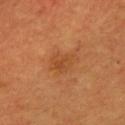Part of a total-body skin-imaging series; this lesion was reviewed on a skin check and was not flagged for biopsy. Automated tile analysis of the lesion measured an average lesion color of about L≈43 a*≈24 b*≈37 (CIELAB), roughly 6 lightness units darker than nearby skin, and a lesion-to-skin contrast of about 5 (normalized; higher = more distinct). The software also gave an automated nevus-likeness rating near 0 out of 100 and a detector confidence of about 100 out of 100 that the crop contains a lesion. The lesion's longest dimension is about 2.5 mm. A male subject approximately 60 years of age. Captured under cross-polarized illumination. The lesion is on the chest. A 15 mm close-up tile from a total-body photography series done for melanoma screening.